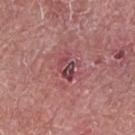anatomic site = the right forearm; subject = female, aged 58–62; image source = ~15 mm crop, total-body skin-cancer survey.